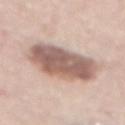Clinical impression: Recorded during total-body skin imaging; not selected for excision or biopsy.